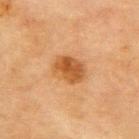Background:
Approximately 4 mm at its widest. Captured under cross-polarized illumination. A female subject, aged around 80. A 15 mm close-up extracted from a 3D total-body photography capture. From the upper back.